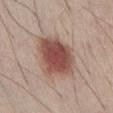| feature | finding |
|---|---|
| workup | imaged on a skin check; not biopsied |